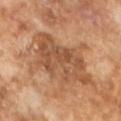biopsy status = catalogued during a skin exam; not biopsied | lesion size = ≈7.5 mm | tile lighting = cross-polarized | image = ~15 mm crop, total-body skin-cancer survey | patient = male, approximately 65 years of age.